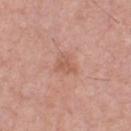The lesion was tiled from a total-body skin photograph and was not biopsied.
The subject is a male in their mid- to late 40s.
The lesion is on the chest.
The lesion-visualizer software estimated a lesion area of about 4 mm², an eccentricity of roughly 0.65, and two-axis asymmetry of about 0.4. And it measured an average lesion color of about L≈57 a*≈23 b*≈30 (CIELAB) and roughly 7 lightness units darker than nearby skin. It also reported a within-lesion color-variation index near 2/10 and a peripheral color-asymmetry measure near 0.5. And it measured an automated nevus-likeness rating near 0 out of 100 and a lesion-detection confidence of about 100/100.
A 15 mm close-up tile from a total-body photography series done for melanoma screening.
This is a white-light tile.
About 2.5 mm across.